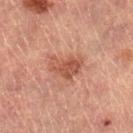Recorded during total-body skin imaging; not selected for excision or biopsy. Located on the right lower leg. A 15 mm close-up tile from a total-body photography series done for melanoma screening. Approximately 4 mm at its widest. Captured under cross-polarized illumination. A female subject in their 70s.